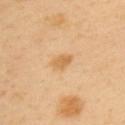Recorded during total-body skin imaging; not selected for excision or biopsy.
The subject is a male in their 40s.
On the left upper arm.
Imaged with cross-polarized lighting.
A region of skin cropped from a whole-body photographic capture, roughly 15 mm wide.